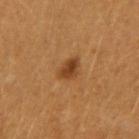No biopsy was performed on this lesion — it was imaged during a full skin examination and was not determined to be concerning.
A female patient aged 28–32.
This image is a 15 mm lesion crop taken from a total-body photograph.
The tile uses cross-polarized illumination.
On the left arm.
Longest diameter approximately 3 mm.